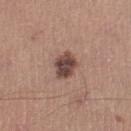Recorded during total-body skin imaging; not selected for excision or biopsy.
A male subject aged 43–47.
A 15 mm close-up extracted from a 3D total-body photography capture.
On the left lower leg.
The recorded lesion diameter is about 3.5 mm.
Automated tile analysis of the lesion measured an outline eccentricity of about 0.65 (0 = round, 1 = elongated) and two-axis asymmetry of about 0.2. And it measured a classifier nevus-likeness of about 30/100 and lesion-presence confidence of about 100/100.
This is a white-light tile.